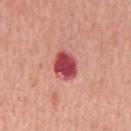Case summary:
- follow-up · catalogued during a skin exam; not biopsied
- tile lighting · white-light illumination
- patient · male, aged around 65
- acquisition · total-body-photography crop, ~15 mm field of view
- location · the mid back